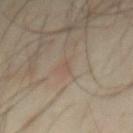Imaged during a routine full-body skin examination; the lesion was not biopsied and no histopathology is available. On the mid back. This is a cross-polarized tile. A male patient roughly 40 years of age. An algorithmic analysis of the crop reported a footprint of about 0.5 mm², an eccentricity of roughly 0.85, and two-axis asymmetry of about 0.4. And it measured a classifier nevus-likeness of about 10/100 and lesion-presence confidence of about 100/100. A close-up tile cropped from a whole-body skin photograph, about 15 mm across.